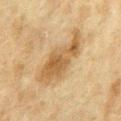Part of a total-body skin-imaging series; this lesion was reviewed on a skin check and was not flagged for biopsy. A 15 mm close-up tile from a total-body photography series done for melanoma screening. A male subject aged approximately 75. From the front of the torso. Measured at roughly 8.5 mm in maximum diameter. Captured under cross-polarized illumination.